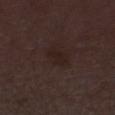automated metrics: an area of roughly 4 mm², an eccentricity of roughly 0.7, and two-axis asymmetry of about 0.3; a mean CIELAB color near L≈19 a*≈13 b*≈16, a lesion–skin lightness drop of about 4, and a lesion-to-skin contrast of about 6 (normalized; higher = more distinct); a within-lesion color-variation index near 1.5/10 and peripheral color asymmetry of about 0.5
imaging modality: ~15 mm tile from a whole-body skin photo
tile lighting: white-light
subject: male, approximately 50 years of age
lesion diameter: about 2.5 mm